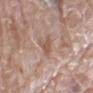follow-up = catalogued during a skin exam; not biopsied
imaging modality = total-body-photography crop, ~15 mm field of view
body site = the leg
tile lighting = white-light
subject = female, approximately 75 years of age